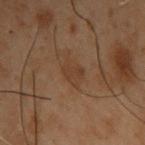Case summary:
* biopsy status · catalogued during a skin exam; not biopsied
* size · ~2.5 mm (longest diameter)
* lighting · cross-polarized illumination
* location · the back
* patient · male, aged approximately 55
* TBP lesion metrics · an area of roughly 3 mm² and a shape eccentricity near 0.85; a lesion color around L≈30 a*≈15 b*≈25 in CIELAB, about 4 CIELAB-L* units darker than the surrounding skin, and a normalized lesion–skin contrast near 5
* acquisition · total-body-photography crop, ~15 mm field of view